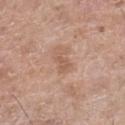Imaged during a routine full-body skin examination; the lesion was not biopsied and no histopathology is available. This image is a 15 mm lesion crop taken from a total-body photograph. A male patient aged 58–62. From the right lower leg.